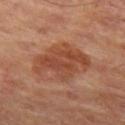Notes:
– diameter · ≈6.5 mm
– tile lighting · cross-polarized illumination
– automated lesion analysis · a mean CIELAB color near L≈45 a*≈24 b*≈32, a lesion–skin lightness drop of about 9, and a normalized border contrast of about 7
– acquisition · ~15 mm tile from a whole-body skin photo
– patient · in their mid-60s
– body site · the left thigh